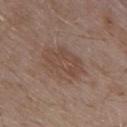Clinical impression: The lesion was tiled from a total-body skin photograph and was not biopsied. Acquisition and patient details: Captured under white-light illumination. A roughly 15 mm field-of-view crop from a total-body skin photograph. About 5.5 mm across. A male subject roughly 55 years of age. On the back.